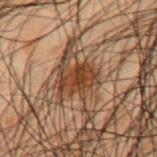{"biopsy_status": "not biopsied; imaged during a skin examination", "patient": {"sex": "male", "age_approx": 50}, "image": {"source": "total-body photography crop", "field_of_view_mm": 15}, "lighting": "cross-polarized", "automated_metrics": {"eccentricity": 0.8, "shape_asymmetry": 0.3, "cielab_L": 30, "cielab_a": 17, "cielab_b": 26, "vs_skin_darker_L": 9.0, "vs_skin_contrast_norm": 10.0}, "site": "upper back"}